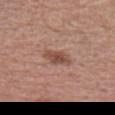Imaged during a routine full-body skin examination; the lesion was not biopsied and no histopathology is available. The recorded lesion diameter is about 3.5 mm. The patient is a male about 70 years old. On the chest. Captured under white-light illumination. This image is a 15 mm lesion crop taken from a total-body photograph.